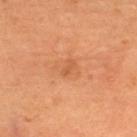notes = imaged on a skin check; not biopsied | acquisition = 15 mm crop, total-body photography | lesion diameter = ≈2 mm | lighting = cross-polarized illumination | patient = female, roughly 35 years of age | site = the upper back.